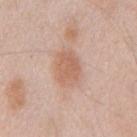Q: Where on the body is the lesion?
A: the chest
Q: How large is the lesion?
A: ~4.5 mm (longest diameter)
Q: What is the imaging modality?
A: 15 mm crop, total-body photography
Q: What did automated image analysis measure?
A: an average lesion color of about L≈62 a*≈20 b*≈30 (CIELAB) and a lesion–skin lightness drop of about 9; a classifier nevus-likeness of about 75/100 and a lesion-detection confidence of about 100/100
Q: Who is the patient?
A: male, aged 43 to 47